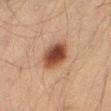Clinical impression:
The lesion was photographed on a routine skin check and not biopsied; there is no pathology result.
Acquisition and patient details:
A roughly 15 mm field-of-view crop from a total-body skin photograph. The recorded lesion diameter is about 4.5 mm. A male patient in their 60s. The lesion is on the leg. Imaged with cross-polarized lighting.